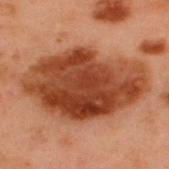- lighting: cross-polarized
- acquisition: ~15 mm crop, total-body skin-cancer survey
- subject: male, roughly 55 years of age
- site: the upper back
- lesion diameter: ~11.5 mm (longest diameter)